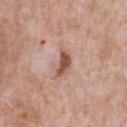biopsy status: no biopsy performed (imaged during a skin exam).